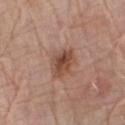follow-up: total-body-photography surveillance lesion; no biopsy
imaging modality: 15 mm crop, total-body photography
subject: male, aged 78 to 82
diameter: about 4 mm
image-analysis metrics: a mean CIELAB color near L≈48 a*≈21 b*≈29, about 11 CIELAB-L* units darker than the surrounding skin, and a normalized lesion–skin contrast near 8.5; an automated nevus-likeness rating near 80 out of 100 and a lesion-detection confidence of about 100/100
location: the right forearm